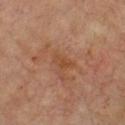Imaged during a routine full-body skin examination; the lesion was not biopsied and no histopathology is available.
A male patient, approximately 70 years of age.
The lesion is on the chest.
Longest diameter approximately 2.5 mm.
A roughly 15 mm field-of-view crop from a total-body skin photograph.
Captured under cross-polarized illumination.